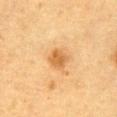<tbp_lesion>
<biopsy_status>not biopsied; imaged during a skin examination</biopsy_status>
<lighting>cross-polarized</lighting>
<lesion_size>
  <long_diameter_mm_approx>2.5</long_diameter_mm_approx>
</lesion_size>
<automated_metrics>
  <color_variation_0_10>2.0</color_variation_0_10>
  <peripheral_color_asymmetry>0.5</peripheral_color_asymmetry>
  <nevus_likeness_0_100>80</nevus_likeness_0_100>
  <lesion_detection_confidence_0_100>100</lesion_detection_confidence_0_100>
</automated_metrics>
<site>abdomen</site>
<image>
  <source>total-body photography crop</source>
  <field_of_view_mm>15</field_of_view_mm>
</image>
<patient>
  <sex>male</sex>
  <age_approx>85+</age_approx>
</patient>
</tbp_lesion>